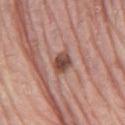Notes:
– notes: imaged on a skin check; not biopsied
– size: ≈2.5 mm
– subject: male, aged 78 to 82
– image source: ~15 mm crop, total-body skin-cancer survey
– location: the leg